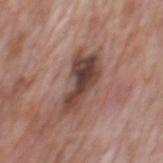workup = total-body-photography surveillance lesion; no biopsy | lesion diameter = about 6.5 mm | anatomic site = the mid back | tile lighting = white-light | image source = ~15 mm crop, total-body skin-cancer survey | subject = male, aged 68 to 72.